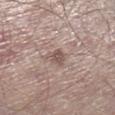Captured during whole-body skin photography for melanoma surveillance; the lesion was not biopsied.
On the leg.
A male subject, roughly 65 years of age.
A 15 mm close-up tile from a total-body photography series done for melanoma screening.